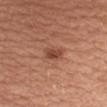notes = total-body-photography surveillance lesion; no biopsy | diameter = about 3 mm | subject = female, aged 53–57 | anatomic site = the left upper arm | image = ~15 mm tile from a whole-body skin photo | tile lighting = white-light illumination.